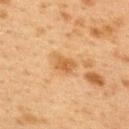{"biopsy_status": "not biopsied; imaged during a skin examination", "image": {"source": "total-body photography crop", "field_of_view_mm": 15}, "site": "upper back", "patient": {"sex": "female", "age_approx": 40}}